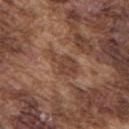Q: Is there a histopathology result?
A: no biopsy performed (imaged during a skin exam)
Q: Who is the patient?
A: male, approximately 75 years of age
Q: Lesion size?
A: ≈4 mm
Q: What is the imaging modality?
A: total-body-photography crop, ~15 mm field of view
Q: Illumination type?
A: white-light
Q: Where on the body is the lesion?
A: the upper back